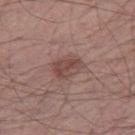Case summary:
• biopsy status: catalogued during a skin exam; not biopsied
• imaging modality: ~15 mm tile from a whole-body skin photo
• lesion size: about 3.5 mm
• tile lighting: white-light illumination
• site: the right thigh
• subject: male, aged 53–57